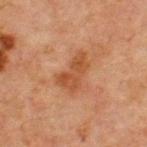Q: Is there a histopathology result?
A: no biopsy performed (imaged during a skin exam)
Q: Lesion location?
A: the chest
Q: Who is the patient?
A: male, approximately 65 years of age
Q: What is the imaging modality?
A: 15 mm crop, total-body photography